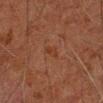Clinical impression:
No biopsy was performed on this lesion — it was imaged during a full skin examination and was not determined to be concerning.
Background:
A male subject, roughly 60 years of age. The recorded lesion diameter is about 2.5 mm. Captured under cross-polarized illumination. Cropped from a whole-body photographic skin survey; the tile spans about 15 mm. The lesion is located on the left arm.